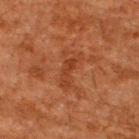workup: no biopsy performed (imaged during a skin exam)
image-analysis metrics: a footprint of about 7.5 mm², a shape eccentricity near 0.9, and a shape-asymmetry score of about 0.3 (0 = symmetric)
imaging modality: ~15 mm crop, total-body skin-cancer survey
illumination: cross-polarized
site: the upper back
lesion size: ≈4.5 mm
patient: male, approximately 60 years of age